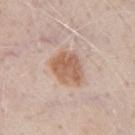workup: catalogued during a skin exam; not biopsied | image: ~15 mm tile from a whole-body skin photo | location: the right upper arm | subject: male, aged approximately 70.